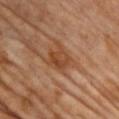This lesion was catalogued during total-body skin photography and was not selected for biopsy. On the chest. A female subject, roughly 65 years of age. This image is a 15 mm lesion crop taken from a total-body photograph. Approximately 3 mm at its widest.